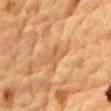Assessment:
This lesion was catalogued during total-body skin photography and was not selected for biopsy.
Acquisition and patient details:
A male subject aged around 85 or older. A 15 mm crop from a total-body photograph taken for skin-cancer surveillance. The lesion is located on the front of the torso. Measured at roughly 3 mm in maximum diameter. Captured under cross-polarized illumination.